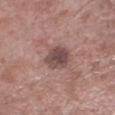biopsy status — imaged on a skin check; not biopsied